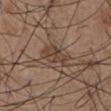Impression:
Captured during whole-body skin photography for melanoma surveillance; the lesion was not biopsied.
Background:
Measured at roughly 4 mm in maximum diameter. The subject is a male aged 53–57. Captured under white-light illumination. The lesion is located on the abdomen. A region of skin cropped from a whole-body photographic capture, roughly 15 mm wide. Automated image analysis of the tile measured an eccentricity of roughly 0.75 and a symmetry-axis asymmetry near 0.35. And it measured a nevus-likeness score of about 5/100 and a detector confidence of about 95 out of 100 that the crop contains a lesion.